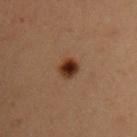Clinical impression: Imaged during a routine full-body skin examination; the lesion was not biopsied and no histopathology is available. Context: Cropped from a whole-body photographic skin survey; the tile spans about 15 mm. Located on the right upper arm. A female patient, aged 38 to 42.